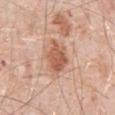A region of skin cropped from a whole-body photographic capture, roughly 15 mm wide.
An algorithmic analysis of the crop reported a footprint of about 9 mm², a shape eccentricity near 0.7, and a shape-asymmetry score of about 0.25 (0 = symmetric). The analysis additionally found a border-irregularity index near 3/10, a within-lesion color-variation index near 3.5/10, and a peripheral color-asymmetry measure near 1.
The patient is a male aged approximately 80.
Approximately 4 mm at its widest.
Captured under white-light illumination.
From the abdomen.